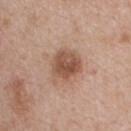Recorded during total-body skin imaging; not selected for excision or biopsy.
Measured at roughly 4.5 mm in maximum diameter.
A 15 mm crop from a total-body photograph taken for skin-cancer surveillance.
A female patient aged approximately 45.
The total-body-photography lesion software estimated a footprint of about 11 mm² and a shape-asymmetry score of about 0.25 (0 = symmetric). The software also gave a border-irregularity index near 2.5/10 and a peripheral color-asymmetry measure near 2. The software also gave a classifier nevus-likeness of about 55/100.
Located on the upper back.
Imaged with white-light lighting.